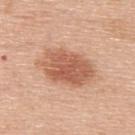Clinical impression: Recorded during total-body skin imaging; not selected for excision or biopsy. Background: A 15 mm crop from a total-body photograph taken for skin-cancer surveillance. An algorithmic analysis of the crop reported a nevus-likeness score of about 90/100 and a lesion-detection confidence of about 100/100. This is a white-light tile. Approximately 7 mm at its widest. A male patient in their 50s. Located on the upper back.